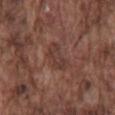patient: male, aged approximately 75 | automated lesion analysis: an area of roughly 8 mm², an outline eccentricity of about 0.85 (0 = round, 1 = elongated), and two-axis asymmetry of about 0.4; internal color variation of about 2 on a 0–10 scale; an automated nevus-likeness rating near 0 out of 100 and lesion-presence confidence of about 85/100 | tile lighting: white-light | anatomic site: the mid back | imaging modality: ~15 mm crop, total-body skin-cancer survey.